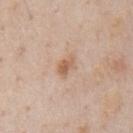Assessment:
Imaged during a routine full-body skin examination; the lesion was not biopsied and no histopathology is available.